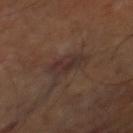biopsy_status: not biopsied; imaged during a skin examination
patient:
  age_approx: 65
lighting: cross-polarized
image:
  source: total-body photography crop
  field_of_view_mm: 15
site: right thigh